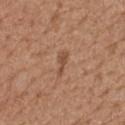This lesion was catalogued during total-body skin photography and was not selected for biopsy. A female subject aged approximately 70. From the upper back. A roughly 15 mm field-of-view crop from a total-body skin photograph.